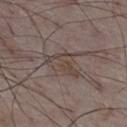Notes:
- body site: the leg
- image: ~15 mm tile from a whole-body skin photo
- patient: male, aged around 75
- tile lighting: white-light illumination
- diameter: ≈5 mm
- TBP lesion metrics: an average lesion color of about L≈43 a*≈12 b*≈20 (CIELAB) and a normalized lesion–skin contrast near 6; a border-irregularity index near 6.5/10, a color-variation rating of about 3.5/10, and radial color variation of about 1